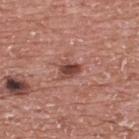  patient:
    sex: male
    age_approx: 70
  automated_metrics:
    area_mm2_approx: 4.5
    eccentricity: 0.7
    shape_asymmetry: 0.3
    cielab_L: 45
    cielab_a: 25
    cielab_b: 25
    vs_skin_darker_L: 13.0
    vs_skin_contrast_norm: 9.0
    border_irregularity_0_10: 3.0
    color_variation_0_10: 3.0
    peripheral_color_asymmetry: 1.0
  lighting: white-light
  image:
    source: total-body photography crop
    field_of_view_mm: 15
  site: upper back